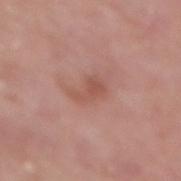Part of a total-body skin-imaging series; this lesion was reviewed on a skin check and was not flagged for biopsy.
A female subject, aged around 65.
The tile uses white-light illumination.
From the front of the torso.
The recorded lesion diameter is about 3.5 mm.
A close-up tile cropped from a whole-body skin photograph, about 15 mm across.